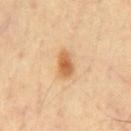<case>
  <biopsy_status>not biopsied; imaged during a skin examination</biopsy_status>
  <site>chest</site>
  <image>
    <source>total-body photography crop</source>
    <field_of_view_mm>15</field_of_view_mm>
  </image>
  <patient>
    <sex>male</sex>
    <age_approx>60</age_approx>
  </patient>
  <lesion_size>
    <long_diameter_mm_approx>3.5</long_diameter_mm_approx>
  </lesion_size>
  <lighting>cross-polarized</lighting>
</case>